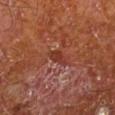This lesion was catalogued during total-body skin photography and was not selected for biopsy. The lesion is on the leg. The lesion's longest dimension is about 3 mm. A close-up tile cropped from a whole-body skin photograph, about 15 mm across. Captured under cross-polarized illumination. Automated tile analysis of the lesion measured a lesion color around L≈35 a*≈27 b*≈30 in CIELAB, about 7 CIELAB-L* units darker than the surrounding skin, and a normalized lesion–skin contrast near 7. And it measured a border-irregularity rating of about 3/10 and radial color variation of about 0.5. A male patient about 65 years old.